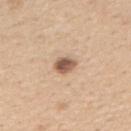| feature | finding |
|---|---|
| follow-up | total-body-photography surveillance lesion; no biopsy |
| site | the right forearm |
| image | 15 mm crop, total-body photography |
| subject | female, about 40 years old |
| lighting | white-light |
| size | ~2.5 mm (longest diameter) |